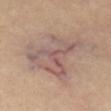This is a cross-polarized tile. The total-body-photography lesion software estimated a lesion color around L≈57 a*≈17 b*≈23 in CIELAB and a normalized border contrast of about 6.5. Cropped from a whole-body photographic skin survey; the tile spans about 15 mm. The recorded lesion diameter is about 7.5 mm. On the abdomen.